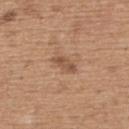Impression:
Captured during whole-body skin photography for melanoma surveillance; the lesion was not biopsied.
Image and clinical context:
A male subject, aged 63–67. Cropped from a whole-body photographic skin survey; the tile spans about 15 mm. The lesion is on the upper back.